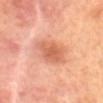notes=imaged on a skin check; not biopsied
lesion diameter=about 5.5 mm
imaging modality=~15 mm crop, total-body skin-cancer survey
patient=female, about 60 years old
location=the mid back
tile lighting=cross-polarized